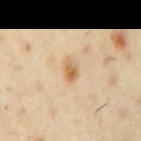Q: Is there a histopathology result?
A: catalogued during a skin exam; not biopsied
Q: What did automated image analysis measure?
A: an area of roughly 3.5 mm², an outline eccentricity of about 0.8 (0 = round, 1 = elongated), and a shape-asymmetry score of about 0.25 (0 = symmetric); a border-irregularity rating of about 2.5/10, a color-variation rating of about 3.5/10, and radial color variation of about 1
Q: How large is the lesion?
A: ~2.5 mm (longest diameter)
Q: How was this image acquired?
A: ~15 mm crop, total-body skin-cancer survey
Q: How was the tile lit?
A: cross-polarized
Q: Patient demographics?
A: male, aged approximately 55
Q: Where on the body is the lesion?
A: the right upper arm